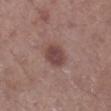follow-up: total-body-photography surveillance lesion; no biopsy
patient: male, aged around 60
location: the right lower leg
acquisition: 15 mm crop, total-body photography